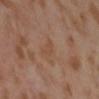{"biopsy_status": "not biopsied; imaged during a skin examination", "site": "abdomen", "patient": {"sex": "female", "age_approx": 55}, "image": {"source": "total-body photography crop", "field_of_view_mm": 15}}